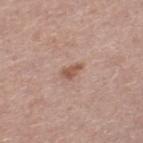notes: catalogued during a skin exam; not biopsied
TBP lesion metrics: roughly 10 lightness units darker than nearby skin and a normalized lesion–skin contrast near 7.5; border irregularity of about 2.5 on a 0–10 scale
location: the right lower leg
lesion size: ≈2.5 mm
image: total-body-photography crop, ~15 mm field of view
tile lighting: white-light
patient: male, about 65 years old